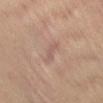Captured during whole-body skin photography for melanoma surveillance; the lesion was not biopsied.
The lesion is located on the abdomen.
The lesion's longest dimension is about 3 mm.
A roughly 15 mm field-of-view crop from a total-body skin photograph.
A male patient roughly 60 years of age.
Automated tile analysis of the lesion measured roughly 6 lightness units darker than nearby skin and a lesion-to-skin contrast of about 4.5 (normalized; higher = more distinct). The software also gave a within-lesion color-variation index near 0/10 and radial color variation of about 0. It also reported a nevus-likeness score of about 0/100 and a lesion-detection confidence of about 100/100.